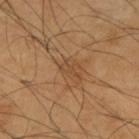Clinical impression: Imaged during a routine full-body skin examination; the lesion was not biopsied and no histopathology is available. Clinical summary: The lesion is on the right upper arm. Captured under cross-polarized illumination. A male patient in their mid-60s. A 15 mm crop from a total-body photograph taken for skin-cancer surveillance. Longest diameter approximately 2.5 mm.